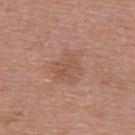Imaged during a routine full-body skin examination; the lesion was not biopsied and no histopathology is available. Automated image analysis of the tile measured a lesion–skin lightness drop of about 6 and a normalized border contrast of about 4.5. A female patient, approximately 50 years of age. A lesion tile, about 15 mm wide, cut from a 3D total-body photograph. The lesion is on the upper back. Approximately 5 mm at its widest. Imaged with white-light lighting.